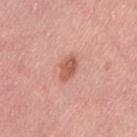<tbp_lesion>
<biopsy_status>not biopsied; imaged during a skin examination</biopsy_status>
<patient>
  <sex>female</sex>
  <age_approx>30</age_approx>
</patient>
<lesion_size>
  <long_diameter_mm_approx>3.0</long_diameter_mm_approx>
</lesion_size>
<site>left thigh</site>
<image>
  <source>total-body photography crop</source>
  <field_of_view_mm>15</field_of_view_mm>
</image>
</tbp_lesion>